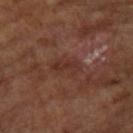<record>
  <biopsy_status>not biopsied; imaged during a skin examination</biopsy_status>
  <patient>
    <sex>male</sex>
    <age_approx>65</age_approx>
  </patient>
  <lesion_size>
    <long_diameter_mm_approx>3.5</long_diameter_mm_approx>
  </lesion_size>
  <site>arm</site>
  <automated_metrics>
    <area_mm2_approx>4.0</area_mm2_approx>
    <eccentricity>0.9</eccentricity>
    <shape_asymmetry>0.35</shape_asymmetry>
    <cielab_L>30</cielab_L>
    <cielab_a>20</cielab_a>
    <cielab_b>23</cielab_b>
    <vs_skin_darker_L>6.0</vs_skin_darker_L>
    <vs_skin_contrast_norm>6.0</vs_skin_contrast_norm>
    <border_irregularity_0_10>4.5</border_irregularity_0_10>
    <color_variation_0_10>1.5</color_variation_0_10>
    <peripheral_color_asymmetry>0.5</peripheral_color_asymmetry>
    <lesion_detection_confidence_0_100>90</lesion_detection_confidence_0_100>
  </automated_metrics>
  <image>
    <source>total-body photography crop</source>
    <field_of_view_mm>15</field_of_view_mm>
  </image>
</record>